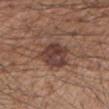A male subject, aged 53–57. A region of skin cropped from a whole-body photographic capture, roughly 15 mm wide. About 4.5 mm across. On the right upper arm. This is a white-light tile.